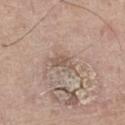An algorithmic analysis of the crop reported a border-irregularity index near 4/10 and a peripheral color-asymmetry measure near 0.5. The analysis additionally found a classifier nevus-likeness of about 0/100 and a detector confidence of about 95 out of 100 that the crop contains a lesion.
The recorded lesion diameter is about 3 mm.
A male patient aged approximately 75.
On the left thigh.
This is a white-light tile.
A 15 mm crop from a total-body photograph taken for skin-cancer surveillance.